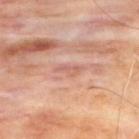| field | value |
|---|---|
| workup | total-body-photography surveillance lesion; no biopsy |
| image source | total-body-photography crop, ~15 mm field of view |
| lesion size | about 2.5 mm |
| automated lesion analysis | a detector confidence of about 60 out of 100 that the crop contains a lesion |
| body site | the back |
| subject | male, approximately 65 years of age |